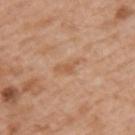Captured during whole-body skin photography for melanoma surveillance; the lesion was not biopsied. A roughly 15 mm field-of-view crop from a total-body skin photograph. Imaged with white-light lighting. A male patient, in their mid- to late 60s. Located on the upper back. The lesion's longest dimension is about 3 mm. Automated tile analysis of the lesion measured a footprint of about 2.5 mm², a shape eccentricity near 0.95, and two-axis asymmetry of about 0.6. The analysis additionally found an average lesion color of about L≈57 a*≈22 b*≈35 (CIELAB) and a lesion–skin lightness drop of about 7. The software also gave border irregularity of about 7 on a 0–10 scale and internal color variation of about 0 on a 0–10 scale. The software also gave a classifier nevus-likeness of about 0/100.